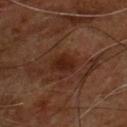Imaged during a routine full-body skin examination; the lesion was not biopsied and no histopathology is available. A male subject in their mid-50s. Captured under cross-polarized illumination. The total-body-photography lesion software estimated a lesion area of about 5 mm² and an eccentricity of roughly 0.65. It also reported a border-irregularity rating of about 2/10, a color-variation rating of about 2/10, and a peripheral color-asymmetry measure near 0.5. And it measured a classifier nevus-likeness of about 20/100 and a detector confidence of about 100 out of 100 that the crop contains a lesion. Measured at roughly 2.5 mm in maximum diameter. The lesion is on the chest. Cropped from a whole-body photographic skin survey; the tile spans about 15 mm.